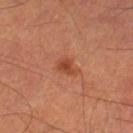An algorithmic analysis of the crop reported an average lesion color of about L≈40 a*≈26 b*≈32 (CIELAB), a lesion–skin lightness drop of about 8, and a normalized lesion–skin contrast near 7.5.
The patient is a male approximately 65 years of age.
The lesion is on the leg.
This is a cross-polarized tile.
Measured at roughly 2.5 mm in maximum diameter.
A 15 mm close-up extracted from a 3D total-body photography capture.